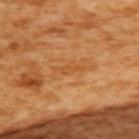{"biopsy_status": "not biopsied; imaged during a skin examination", "automated_metrics": {"cielab_L": 54, "cielab_a": 27, "cielab_b": 45, "vs_skin_darker_L": 6.0, "vs_skin_contrast_norm": 5.0, "color_variation_0_10": 0.0, "peripheral_color_asymmetry": 0.0, "lesion_detection_confidence_0_100": 65}, "site": "upper back", "lighting": "cross-polarized", "patient": {"sex": "female", "age_approx": 55}, "image": {"source": "total-body photography crop", "field_of_view_mm": 15}, "lesion_size": {"long_diameter_mm_approx": 3.5}}